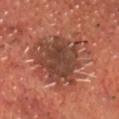Clinical impression:
Part of a total-body skin-imaging series; this lesion was reviewed on a skin check and was not flagged for biopsy.
Background:
The subject is a male approximately 70 years of age. The lesion is located on the chest. A lesion tile, about 15 mm wide, cut from a 3D total-body photograph. Automated image analysis of the tile measured a shape eccentricity near 0.5 and two-axis asymmetry of about 0.4. And it measured border irregularity of about 6 on a 0–10 scale, a color-variation rating of about 6/10, and peripheral color asymmetry of about 2. Longest diameter approximately 8.5 mm. Captured under cross-polarized illumination.